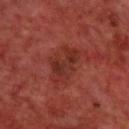biopsy status = imaged on a skin check; not biopsied
body site = the back
patient = male, aged approximately 70
image source = 15 mm crop, total-body photography